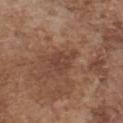  lesion_size:
    long_diameter_mm_approx: 3.0
  lighting: white-light
  site: chest
  image:
    source: total-body photography crop
    field_of_view_mm: 15
  patient:
    sex: male
    age_approx: 75